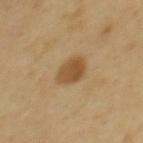Imaged with cross-polarized lighting. A female patient, in their 60s. The lesion is located on the back. Cropped from a total-body skin-imaging series; the visible field is about 15 mm. An algorithmic analysis of the crop reported a lesion area of about 7 mm² and a shape-asymmetry score of about 0.15 (0 = symmetric). It also reported a mean CIELAB color near L≈51 a*≈17 b*≈38, roughly 11 lightness units darker than nearby skin, and a normalized border contrast of about 8.5. And it measured a classifier nevus-likeness of about 95/100 and a lesion-detection confidence of about 100/100. The lesion's longest dimension is about 3.5 mm.